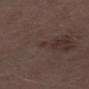This lesion was catalogued during total-body skin photography and was not selected for biopsy.
An algorithmic analysis of the crop reported an average lesion color of about L≈31 a*≈14 b*≈18 (CIELAB), roughly 5 lightness units darker than nearby skin, and a lesion-to-skin contrast of about 5.5 (normalized; higher = more distinct). It also reported border irregularity of about 3 on a 0–10 scale, internal color variation of about 0 on a 0–10 scale, and peripheral color asymmetry of about 0. The software also gave a classifier nevus-likeness of about 0/100.
Cropped from a total-body skin-imaging series; the visible field is about 15 mm.
Longest diameter approximately 1 mm.
Located on the left lower leg.
A male patient aged 68 to 72.
This is a white-light tile.